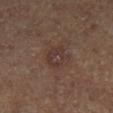A close-up tile cropped from a whole-body skin photograph, about 15 mm across. Located on the leg. The subject is a male in their mid- to late 60s.